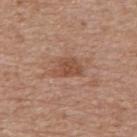Case summary:
– workup: imaged on a skin check; not biopsied
– illumination: white-light illumination
– automated metrics: a lesion area of about 6 mm², an eccentricity of roughly 0.7, and a symmetry-axis asymmetry near 0.4; a nevus-likeness score of about 5/100 and a detector confidence of about 100 out of 100 that the crop contains a lesion
– location: the upper back
– lesion diameter: ≈3.5 mm
– subject: male, aged approximately 80
– image source: total-body-photography crop, ~15 mm field of view